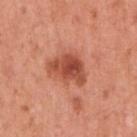The lesion was photographed on a routine skin check and not biopsied; there is no pathology result.
This is a white-light tile.
Longest diameter approximately 4.5 mm.
Automated tile analysis of the lesion measured an area of roughly 11 mm², an outline eccentricity of about 0.7 (0 = round, 1 = elongated), and a symmetry-axis asymmetry near 0.35. It also reported an average lesion color of about L≈51 a*≈31 b*≈34 (CIELAB). It also reported a classifier nevus-likeness of about 85/100 and lesion-presence confidence of about 100/100.
A female patient, roughly 50 years of age.
A 15 mm close-up tile from a total-body photography series done for melanoma screening.
Located on the right upper arm.